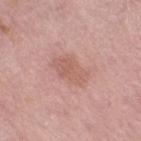Q: Patient demographics?
A: female, about 70 years old
Q: What is the imaging modality?
A: 15 mm crop, total-body photography
Q: How large is the lesion?
A: ≈4 mm
Q: What is the anatomic site?
A: the leg